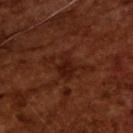follow-up=total-body-photography surveillance lesion; no biopsy
imaging modality=15 mm crop, total-body photography
automated lesion analysis=a mean CIELAB color near L≈21 a*≈22 b*≈25, about 5 CIELAB-L* units darker than the surrounding skin, and a normalized border contrast of about 6.5; a border-irregularity index near 7.5/10 and a color-variation rating of about 3.5/10
subject=male, aged 63 to 67